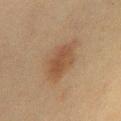Q: Was this lesion biopsied?
A: no biopsy performed (imaged during a skin exam)
Q: What kind of image is this?
A: ~15 mm crop, total-body skin-cancer survey
Q: What is the anatomic site?
A: the front of the torso
Q: Illumination type?
A: cross-polarized illumination
Q: How large is the lesion?
A: ~5.5 mm (longest diameter)
Q: Patient demographics?
A: female, in their 50s